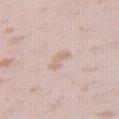<record>
  <biopsy_status>not biopsied; imaged during a skin examination</biopsy_status>
  <lighting>white-light</lighting>
  <image>
    <source>total-body photography crop</source>
    <field_of_view_mm>15</field_of_view_mm>
  </image>
  <site>right thigh</site>
  <patient>
    <sex>female</sex>
    <age_approx>25</age_approx>
  </patient>
</record>